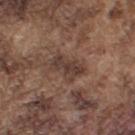follow-up = total-body-photography surveillance lesion; no biopsy | tile lighting = white-light | subject = male, aged 73 to 77 | location = the left upper arm | acquisition = 15 mm crop, total-body photography | lesion diameter = about 4 mm.